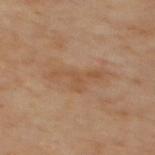workup = imaged on a skin check; not biopsied
illumination = cross-polarized illumination
acquisition = total-body-photography crop, ~15 mm field of view
body site = the mid back
size = about 6 mm